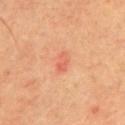Assessment: This lesion was catalogued during total-body skin photography and was not selected for biopsy. Clinical summary: A 15 mm close-up extracted from a 3D total-body photography capture. Longest diameter approximately 2.5 mm. From the chest. A male subject, roughly 70 years of age. This is a cross-polarized tile.